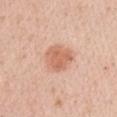Imaged during a routine full-body skin examination; the lesion was not biopsied and no histopathology is available. The tile uses white-light illumination. A roughly 15 mm field-of-view crop from a total-body skin photograph. From the right upper arm. A male subject, aged around 55. Approximately 3.5 mm at its widest.